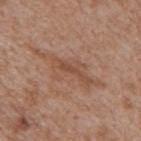biopsy status: imaged on a skin check; not biopsied | image source: ~15 mm crop, total-body skin-cancer survey | anatomic site: the back | patient: male, in their mid-60s.